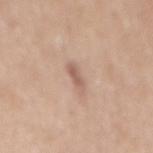<case>
<biopsy_status>not biopsied; imaged during a skin examination</biopsy_status>
<site>mid back</site>
<image>
  <source>total-body photography crop</source>
  <field_of_view_mm>15</field_of_view_mm>
</image>
<patient>
  <sex>female</sex>
  <age_approx>40</age_approx>
</patient>
</case>